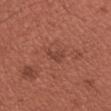- biopsy status · catalogued during a skin exam; not biopsied
- lesion size · ~2.5 mm (longest diameter)
- patient · male, about 35 years old
- imaging modality · total-body-photography crop, ~15 mm field of view
- site · the chest
- automated lesion analysis · a nevus-likeness score of about 0/100 and lesion-presence confidence of about 100/100
- lighting · white-light